The lesion is on the chest · the tile uses white-light illumination · longest diameter approximately 2 mm · Automated image analysis of the tile measured a footprint of about 1.5 mm², an outline eccentricity of about 0.85 (0 = round, 1 = elongated), and a shape-asymmetry score of about 0.55 (0 = symmetric). The software also gave a mean CIELAB color near L≈54 a*≈25 b*≈34, about 8 CIELAB-L* units darker than the surrounding skin, and a normalized border contrast of about 5.5. The analysis additionally found a border-irregularity index near 5/10, internal color variation of about 0 on a 0–10 scale, and radial color variation of about 0 · the patient is a female about 70 years old · a close-up tile cropped from a whole-body skin photograph, about 15 mm across:
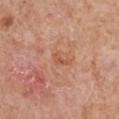diagnosis: a superficial basal cell carcinoma — a malignant skin lesion.